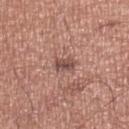Imaged during a routine full-body skin examination; the lesion was not biopsied and no histopathology is available. A lesion tile, about 15 mm wide, cut from a 3D total-body photograph. On the left lower leg. This is a white-light tile. A male subject aged 68–72. Measured at roughly 2.5 mm in maximum diameter.